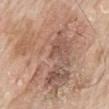The lesion was tiled from a total-body skin photograph and was not biopsied.
On the mid back.
A region of skin cropped from a whole-body photographic capture, roughly 15 mm wide.
The subject is a male roughly 65 years of age.